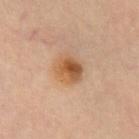  biopsy_status: not biopsied; imaged during a skin examination
  lesion_size:
    long_diameter_mm_approx: 3.5
  automated_metrics:
    cielab_L: 57
    cielab_a: 22
    cielab_b: 38
    vs_skin_contrast_norm: 9.0
    nevus_likeness_0_100: 95
    lesion_detection_confidence_0_100: 100
  site: front of the torso
  image:
    source: total-body photography crop
    field_of_view_mm: 15
  lighting: cross-polarized
  patient:
    sex: female
    age_approx: 60